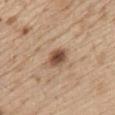Q: Was this lesion biopsied?
A: total-body-photography surveillance lesion; no biopsy
Q: How was this image acquired?
A: ~15 mm crop, total-body skin-cancer survey
Q: Where on the body is the lesion?
A: the front of the torso
Q: What did automated image analysis measure?
A: a lesion color around L≈50 a*≈19 b*≈30 in CIELAB, about 15 CIELAB-L* units darker than the surrounding skin, and a normalized lesion–skin contrast near 10.5; border irregularity of about 2 on a 0–10 scale and a within-lesion color-variation index near 4.5/10
Q: Patient demographics?
A: male, about 70 years old